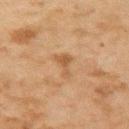Findings:
- workup — imaged on a skin check; not biopsied
- lighting — cross-polarized
- acquisition — ~15 mm crop, total-body skin-cancer survey
- patient — male, roughly 45 years of age
- body site — the left upper arm
- size — about 3.5 mm